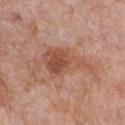Recorded during total-body skin imaging; not selected for excision or biopsy. On the chest. A 15 mm close-up extracted from a 3D total-body photography capture. Longest diameter approximately 6.5 mm. The tile uses white-light illumination. The subject is a female roughly 70 years of age.